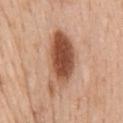The lesion-visualizer software estimated border irregularity of about 4 on a 0–10 scale, a within-lesion color-variation index near 8.5/10, and a peripheral color-asymmetry measure near 3. A female patient about 50 years old. Located on the mid back. Cropped from a whole-body photographic skin survey; the tile spans about 15 mm. Captured under white-light illumination. The lesion's longest dimension is about 8 mm.